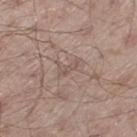Clinical impression:
The lesion was tiled from a total-body skin photograph and was not biopsied.
Background:
This is a white-light tile. A male subject, in their mid-50s. The total-body-photography lesion software estimated an area of roughly 2 mm² and a symmetry-axis asymmetry near 0.55. And it measured a lesion–skin lightness drop of about 7 and a lesion-to-skin contrast of about 5 (normalized; higher = more distinct). It also reported an automated nevus-likeness rating near 0 out of 100 and a lesion-detection confidence of about 65/100. Measured at roughly 2.5 mm in maximum diameter. A 15 mm close-up extracted from a 3D total-body photography capture. The lesion is on the left thigh.